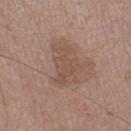Clinical impression:
The lesion was photographed on a routine skin check and not biopsied; there is no pathology result.
Context:
Located on the right thigh. About 5 mm across. A male patient aged around 75. A 15 mm crop from a total-body photograph taken for skin-cancer surveillance.